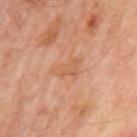Findings:
– biopsy status — no biopsy performed (imaged during a skin exam)
– location — the left upper arm
– automated metrics — a mean CIELAB color near L≈57 a*≈22 b*≈34, about 6 CIELAB-L* units darker than the surrounding skin, and a lesion-to-skin contrast of about 5 (normalized; higher = more distinct)
– tile lighting — cross-polarized
– patient — male, in their mid- to late 60s
– lesion size — ~4 mm (longest diameter)
– acquisition — total-body-photography crop, ~15 mm field of view